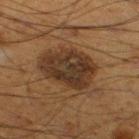workup: imaged on a skin check; not biopsied | subject: male, aged approximately 60 | body site: the right thigh | imaging modality: total-body-photography crop, ~15 mm field of view | size: ~7.5 mm (longest diameter) | image-analysis metrics: an outline eccentricity of about 0.75 (0 = round, 1 = elongated) and a symmetry-axis asymmetry near 0.25; a mean CIELAB color near L≈28 a*≈14 b*≈25, roughly 9 lightness units darker than nearby skin, and a normalized lesion–skin contrast near 9.5; a border-irregularity rating of about 3/10, a within-lesion color-variation index near 4.5/10, and radial color variation of about 1.5; a nevus-likeness score of about 35/100 and a lesion-detection confidence of about 95/100 | lighting: cross-polarized illumination.